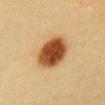No biopsy was performed on this lesion — it was imaged during a full skin examination and was not determined to be concerning. Located on the chest. A roughly 15 mm field-of-view crop from a total-body skin photograph. The patient is a female aged approximately 30.